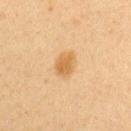  biopsy_status: not biopsied; imaged during a skin examination
  lighting: cross-polarized
  patient:
    sex: female
    age_approx: 40
  site: left upper arm
  image:
    source: total-body photography crop
    field_of_view_mm: 15
  lesion_size:
    long_diameter_mm_approx: 3.0